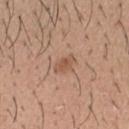No biopsy was performed on this lesion — it was imaged during a full skin examination and was not determined to be concerning.
From the head or neck.
Cropped from a total-body skin-imaging series; the visible field is about 15 mm.
The subject is a male approximately 30 years of age.